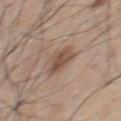| field | value |
|---|---|
| notes | imaged on a skin check; not biopsied |
| illumination | white-light |
| lesion diameter | ~3 mm (longest diameter) |
| patient | male, aged 63 to 67 |
| image | ~15 mm tile from a whole-body skin photo |
| anatomic site | the back |